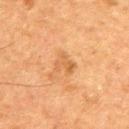Clinical summary:
This is a cross-polarized tile. A lesion tile, about 15 mm wide, cut from a 3D total-body photograph. A male subject, in their 50s. Located on the back.